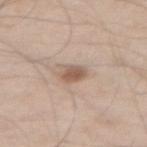notes = total-body-photography surveillance lesion; no biopsy